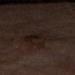<tbp_lesion>
  <biopsy_status>not biopsied; imaged during a skin examination</biopsy_status>
  <automated_metrics>
    <area_mm2_approx>13.0</area_mm2_approx>
    <shape_asymmetry>0.25</shape_asymmetry>
    <cielab_L>15</cielab_L>
    <cielab_a>10</cielab_a>
    <cielab_b>14</cielab_b>
    <vs_skin_darker_L>4.0</vs_skin_darker_L>
    <vs_skin_contrast_norm>6.5</vs_skin_contrast_norm>
    <color_variation_0_10>4.0</color_variation_0_10>
    <peripheral_color_asymmetry>1.0</peripheral_color_asymmetry>
    <nevus_likeness_0_100>0</nevus_likeness_0_100>
  </automated_metrics>
  <image>
    <source>total-body photography crop</source>
    <field_of_view_mm>15</field_of_view_mm>
  </image>
  <site>left thigh</site>
  <patient>
    <sex>male</sex>
    <age_approx>70</age_approx>
  </patient>
  <lesion_size>
    <long_diameter_mm_approx>6.0</long_diameter_mm_approx>
  </lesion_size>
  <lighting>cross-polarized</lighting>
</tbp_lesion>